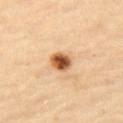Clinical impression:
No biopsy was performed on this lesion — it was imaged during a full skin examination and was not determined to be concerning.
Image and clinical context:
The patient is a female aged around 70. Imaged with cross-polarized lighting. Cropped from a total-body skin-imaging series; the visible field is about 15 mm. The lesion is on the left thigh.